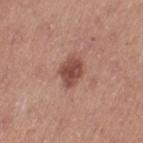This lesion was catalogued during total-body skin photography and was not selected for biopsy. A 15 mm close-up tile from a total-body photography series done for melanoma screening. A female subject, aged around 40. Captured under white-light illumination. The lesion's longest dimension is about 3.5 mm. On the left thigh. The lesion-visualizer software estimated an average lesion color of about L≈47 a*≈24 b*≈25 (CIELAB) and a normalized lesion–skin contrast near 9.